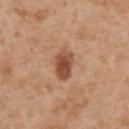Part of a total-body skin-imaging series; this lesion was reviewed on a skin check and was not flagged for biopsy.
Imaged with white-light lighting.
A male patient, approximately 55 years of age.
The lesion is on the upper back.
The lesion's longest dimension is about 3.5 mm.
A 15 mm crop from a total-body photograph taken for skin-cancer surveillance.